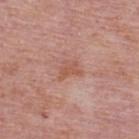Impression:
Part of a total-body skin-imaging series; this lesion was reviewed on a skin check and was not flagged for biopsy.
Background:
From the upper back. The tile uses white-light illumination. A lesion tile, about 15 mm wide, cut from a 3D total-body photograph. The lesion-visualizer software estimated a border-irregularity index near 4.5/10 and a within-lesion color-variation index near 3.5/10. Measured at roughly 2.5 mm in maximum diameter. The subject is a male aged around 75.